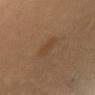Q: Was a biopsy performed?
A: no biopsy performed (imaged during a skin exam)